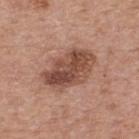follow-up=catalogued during a skin exam; not biopsied
patient=male, aged 78–82
imaging modality=15 mm crop, total-body photography
body site=the upper back
diameter=~6.5 mm (longest diameter)
lighting=white-light